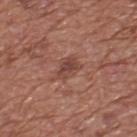acquisition: ~15 mm crop, total-body skin-cancer survey | size: ≈3 mm | illumination: white-light | image-analysis metrics: an average lesion color of about L≈43 a*≈23 b*≈25 (CIELAB), a lesion–skin lightness drop of about 9, and a lesion-to-skin contrast of about 7 (normalized; higher = more distinct); a within-lesion color-variation index near 2.5/10 and a peripheral color-asymmetry measure near 1; an automated nevus-likeness rating near 40 out of 100 and a lesion-detection confidence of about 100/100 | anatomic site: the upper back | patient: male, aged 73 to 77.